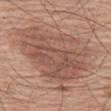The lesion was photographed on a routine skin check and not biopsied; there is no pathology result.
A male subject, about 65 years old.
Measured at roughly 8.5 mm in maximum diameter.
Captured under white-light illumination.
Automated image analysis of the tile measured a lesion color around L≈52 a*≈21 b*≈27 in CIELAB, about 9 CIELAB-L* units darker than the surrounding skin, and a normalized lesion–skin contrast near 6. It also reported border irregularity of about 7 on a 0–10 scale, internal color variation of about 4.5 on a 0–10 scale, and a peripheral color-asymmetry measure near 1.5.
This image is a 15 mm lesion crop taken from a total-body photograph.
On the leg.